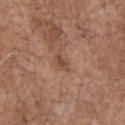Imaged during a routine full-body skin examination; the lesion was not biopsied and no histopathology is available. The tile uses white-light illumination. The patient is a male aged around 70. Cropped from a total-body skin-imaging series; the visible field is about 15 mm. About 2.5 mm across. Located on the chest.